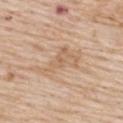Recorded during total-body skin imaging; not selected for excision or biopsy.
The lesion is located on the upper back.
Captured under white-light illumination.
A female patient, approximately 70 years of age.
Cropped from a whole-body photographic skin survey; the tile spans about 15 mm.